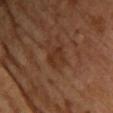Case summary:
• biopsy status: catalogued during a skin exam; not biopsied
• image source: ~15 mm tile from a whole-body skin photo
• anatomic site: the upper back
• patient: female, aged 63 to 67
• lesion diameter: about 2.5 mm
• tile lighting: cross-polarized illumination